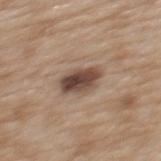A close-up tile cropped from a whole-body skin photograph, about 15 mm across.
Located on the upper back.
The subject is a female aged around 40.